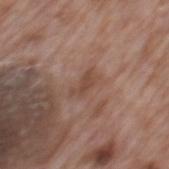Q: Was a biopsy performed?
A: total-body-photography surveillance lesion; no biopsy
Q: Where on the body is the lesion?
A: the mid back
Q: What is the lesion's diameter?
A: about 3 mm
Q: What kind of image is this?
A: 15 mm crop, total-body photography
Q: Who is the patient?
A: male, roughly 70 years of age
Q: How was the tile lit?
A: white-light illumination
Q: Automated lesion metrics?
A: a border-irregularity rating of about 3.5/10, a color-variation rating of about 1.5/10, and peripheral color asymmetry of about 0.5